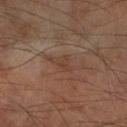Clinical impression:
Imaged during a routine full-body skin examination; the lesion was not biopsied and no histopathology is available.
Image and clinical context:
A 15 mm crop from a total-body photograph taken for skin-cancer surveillance. Located on the right forearm. A male patient aged 68 to 72. Automated image analysis of the tile measured a footprint of about 3.5 mm², an outline eccentricity of about 0.7 (0 = round, 1 = elongated), and a symmetry-axis asymmetry near 0.4. The analysis additionally found a lesion color around L≈39 a*≈18 b*≈26 in CIELAB, about 5 CIELAB-L* units darker than the surrounding skin, and a lesion-to-skin contrast of about 4.5 (normalized; higher = more distinct). This is a cross-polarized tile.